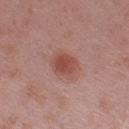The lesion was tiled from a total-body skin photograph and was not biopsied. The lesion's longest dimension is about 3 mm. A lesion tile, about 15 mm wide, cut from a 3D total-body photograph. From the left upper arm. A male patient in their mid-50s.